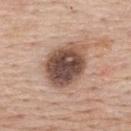Impression: Captured during whole-body skin photography for melanoma surveillance; the lesion was not biopsied. Image and clinical context: The subject is a female aged 63–67. Imaged with white-light lighting. The lesion is on the upper back. Longest diameter approximately 6 mm. A region of skin cropped from a whole-body photographic capture, roughly 15 mm wide. The lesion-visualizer software estimated a border-irregularity index near 1.5/10, a within-lesion color-variation index near 7/10, and radial color variation of about 2.